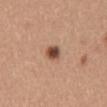Impression:
Captured during whole-body skin photography for melanoma surveillance; the lesion was not biopsied.
Acquisition and patient details:
The lesion-visualizer software estimated a border-irregularity index near 1.5/10, a within-lesion color-variation index near 5/10, and radial color variation of about 1.5. The analysis additionally found a classifier nevus-likeness of about 100/100 and lesion-presence confidence of about 100/100. On the mid back. Measured at roughly 2.5 mm in maximum diameter. A female subject, in their 30s. Captured under white-light illumination. A close-up tile cropped from a whole-body skin photograph, about 15 mm across.